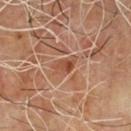{
  "biopsy_status": "not biopsied; imaged during a skin examination",
  "patient": {
    "sex": "male",
    "age_approx": 65
  },
  "automated_metrics": {
    "area_mm2_approx": 4.5,
    "eccentricity": 0.8,
    "shape_asymmetry": 0.4,
    "cielab_L": 47,
    "cielab_a": 23,
    "cielab_b": 31,
    "vs_skin_contrast_norm": 7.0
  },
  "image": {
    "source": "total-body photography crop",
    "field_of_view_mm": 15
  },
  "site": "chest",
  "lighting": "cross-polarized",
  "lesion_size": {
    "long_diameter_mm_approx": 3.0
  }
}